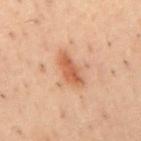The lesion was photographed on a routine skin check and not biopsied; there is no pathology result. The subject is a male aged approximately 55. From the mid back. A lesion tile, about 15 mm wide, cut from a 3D total-body photograph.